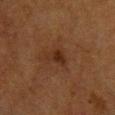{"biopsy_status": "not biopsied; imaged during a skin examination", "site": "chest", "image": {"source": "total-body photography crop", "field_of_view_mm": 15}, "automated_metrics": {"eccentricity": 0.75, "shape_asymmetry": 0.45, "border_irregularity_0_10": 4.0, "peripheral_color_asymmetry": 1.0, "nevus_likeness_0_100": 15}, "patient": {"sex": "female", "age_approx": 55}, "lesion_size": {"long_diameter_mm_approx": 3.0}, "lighting": "cross-polarized"}